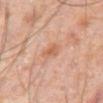image source = ~15 mm crop, total-body skin-cancer survey | tile lighting = cross-polarized | location = the abdomen | subject = male, about 60 years old | image-analysis metrics = a mean CIELAB color near L≈56 a*≈22 b*≈31, roughly 7 lightness units darker than nearby skin, and a normalized border contrast of about 5.5; a border-irregularity rating of about 3/10, a within-lesion color-variation index near 3/10, and peripheral color asymmetry of about 1; a detector confidence of about 100 out of 100 that the crop contains a lesion.